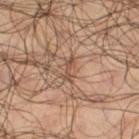follow-up: total-body-photography surveillance lesion; no biopsy
anatomic site: the right thigh
image source: ~15 mm tile from a whole-body skin photo
subject: male, approximately 65 years of age
diameter: ~3 mm (longest diameter)
automated lesion analysis: a lesion color around L≈46 a*≈18 b*≈27 in CIELAB, roughly 9 lightness units darker than nearby skin, and a normalized lesion–skin contrast near 6.5; border irregularity of about 6 on a 0–10 scale and peripheral color asymmetry of about 0